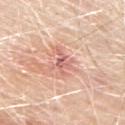The lesion was photographed on a routine skin check and not biopsied; there is no pathology result. An algorithmic analysis of the crop reported an outline eccentricity of about 0.85 (0 = round, 1 = elongated) and two-axis asymmetry of about 0.45. The software also gave a lesion color around L≈64 a*≈26 b*≈27 in CIELAB, about 11 CIELAB-L* units darker than the surrounding skin, and a lesion-to-skin contrast of about 6.5 (normalized; higher = more distinct). And it measured an automated nevus-likeness rating near 0 out of 100. A 15 mm crop from a total-body photograph taken for skin-cancer surveillance. On the back. The patient is a male in their mid- to late 60s. Measured at roughly 3.5 mm in maximum diameter.